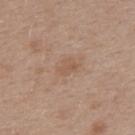<record>
<image>
  <source>total-body photography crop</source>
  <field_of_view_mm>15</field_of_view_mm>
</image>
<lighting>white-light</lighting>
<patient>
  <sex>female</sex>
  <age_approx>40</age_approx>
</patient>
<automated_metrics>
  <shape_asymmetry>0.25</shape_asymmetry>
</automated_metrics>
<lesion_size>
  <long_diameter_mm_approx>2.5</long_diameter_mm_approx>
</lesion_size>
<site>upper back</site>
</record>